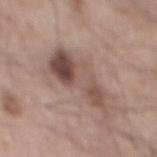Q: Is there a histopathology result?
A: no biopsy performed (imaged during a skin exam)